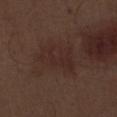  site: right thigh
  lighting: white-light
  image:
    source: total-body photography crop
    field_of_view_mm: 15
  lesion_size:
    long_diameter_mm_approx: 5.0
  patient:
    sex: male
    age_approx: 70